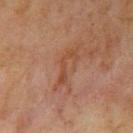The lesion was tiled from a total-body skin photograph and was not biopsied. A 15 mm close-up extracted from a 3D total-body photography capture. From the arm. A female patient, roughly 55 years of age. Captured under cross-polarized illumination. The lesion-visualizer software estimated a mean CIELAB color near L≈41 a*≈21 b*≈30, about 6 CIELAB-L* units darker than the surrounding skin, and a normalized lesion–skin contrast near 6. And it measured a border-irregularity rating of about 8.5/10, a within-lesion color-variation index near 0/10, and peripheral color asymmetry of about 0. It also reported a nevus-likeness score of about 0/100 and a lesion-detection confidence of about 100/100.